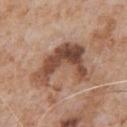Impression: Imaged during a routine full-body skin examination; the lesion was not biopsied and no histopathology is available. Background: The subject is a male aged around 65. Cropped from a whole-body photographic skin survey; the tile spans about 15 mm. Captured under white-light illumination. Longest diameter approximately 7 mm. The lesion-visualizer software estimated an average lesion color of about L≈48 a*≈20 b*≈28 (CIELAB) and a normalized border contrast of about 10. The analysis additionally found border irregularity of about 7.5 on a 0–10 scale and radial color variation of about 2. The lesion is on the front of the torso.